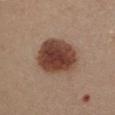biopsy_status: not biopsied; imaged during a skin examination
image:
  source: total-body photography crop
  field_of_view_mm: 15
lesion_size:
  long_diameter_mm_approx: 5.5
site: chest
patient:
  sex: female
  age_approx: 30
lighting: white-light